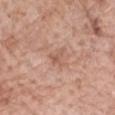Notes:
* anatomic site: the left forearm
* lesion size: ≈2.5 mm
* TBP lesion metrics: an automated nevus-likeness rating near 0 out of 100 and lesion-presence confidence of about 100/100
* image: 15 mm crop, total-body photography
* subject: female, in their 70s
* illumination: white-light